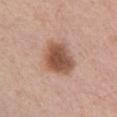notes: no biopsy performed (imaged during a skin exam)
imaging modality: ~15 mm crop, total-body skin-cancer survey
patient: female, about 40 years old
anatomic site: the chest
image-analysis metrics: an outline eccentricity of about 0.6 (0 = round, 1 = elongated) and a shape-asymmetry score of about 0.2 (0 = symmetric); a border-irregularity index near 2/10, a color-variation rating of about 4.5/10, and radial color variation of about 1; an automated nevus-likeness rating near 90 out of 100 and lesion-presence confidence of about 100/100